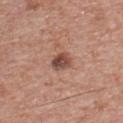This is a white-light tile. A close-up tile cropped from a whole-body skin photograph, about 15 mm across. Approximately 2.5 mm at its widest. From the front of the torso. The total-body-photography lesion software estimated a lesion color around L≈48 a*≈22 b*≈25 in CIELAB, a lesion–skin lightness drop of about 13, and a lesion-to-skin contrast of about 9.5 (normalized; higher = more distinct). The analysis additionally found a border-irregularity index near 1.5/10, a color-variation rating of about 5/10, and a peripheral color-asymmetry measure near 1.5. A male subject about 70 years old.